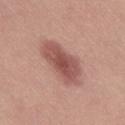  image:
    source: total-body photography crop
    field_of_view_mm: 15
  patient:
    sex: female
    age_approx: 30
  site: leg
  diagnosis:
    histopathology: intradermal melanocytic nevus
    malignancy: benign
    taxonomic_path:
      - Benign
      - Benign melanocytic proliferations
      - Nevus
      - Nevus, NOS, Dermal